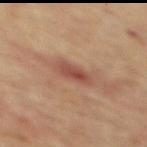Assessment:
Recorded during total-body skin imaging; not selected for excision or biopsy.
Clinical summary:
On the mid back. A male subject, aged approximately 65. A region of skin cropped from a whole-body photographic capture, roughly 15 mm wide.